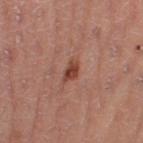• biopsy status: total-body-photography surveillance lesion; no biopsy
• tile lighting: cross-polarized illumination
• size: ≈2.5 mm
• patient: male, approximately 55 years of age
• body site: the leg
• acquisition: ~15 mm crop, total-body skin-cancer survey